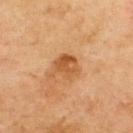follow-up: imaged on a skin check; not biopsied | lesion size: ~3 mm (longest diameter) | automated metrics: a footprint of about 6.5 mm², an eccentricity of roughly 0.5, and a shape-asymmetry score of about 0.2 (0 = symmetric); a lesion color around L≈47 a*≈22 b*≈37 in CIELAB and roughly 9 lightness units darker than nearby skin; a border-irregularity rating of about 2/10, internal color variation of about 4.5 on a 0–10 scale, and peripheral color asymmetry of about 1.5; a detector confidence of about 100 out of 100 that the crop contains a lesion | body site: the upper back | imaging modality: ~15 mm tile from a whole-body skin photo | subject: female, roughly 55 years of age | lighting: cross-polarized.